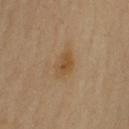follow-up — catalogued during a skin exam; not biopsied | body site — the left upper arm | acquisition — ~15 mm crop, total-body skin-cancer survey | subject — female, aged approximately 70 | tile lighting — cross-polarized.